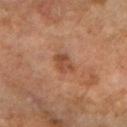| feature | finding |
|---|---|
| patient | female, aged approximately 60 |
| lighting | cross-polarized |
| size | about 3 mm |
| image | total-body-photography crop, ~15 mm field of view |
| anatomic site | the right forearm |